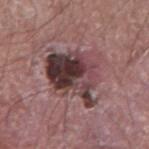{
  "biopsy_status": "not biopsied; imaged during a skin examination",
  "lighting": "white-light",
  "patient": {
    "sex": "male",
    "age_approx": 60
  },
  "image": {
    "source": "total-body photography crop",
    "field_of_view_mm": 15
  },
  "lesion_size": {
    "long_diameter_mm_approx": 6.0
  },
  "site": "left forearm"
}